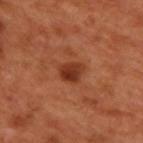This lesion was catalogued during total-body skin photography and was not selected for biopsy. The lesion is on the upper back. A 15 mm close-up tile from a total-body photography series done for melanoma screening. A male subject, roughly 50 years of age.